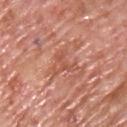Findings:
– workup: catalogued during a skin exam; not biopsied
– size: ≈3.5 mm
– automated metrics: a border-irregularity rating of about 8.5/10, a within-lesion color-variation index near 1.5/10, and radial color variation of about 0
– location: the chest
– acquisition: total-body-photography crop, ~15 mm field of view
– patient: male, in their mid-70s
– tile lighting: white-light